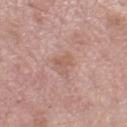follow-up: total-body-photography surveillance lesion; no biopsy
subject: female, approximately 50 years of age
tile lighting: white-light illumination
location: the leg
automated lesion analysis: a mean CIELAB color near L≈58 a*≈21 b*≈26 and a normalized border contrast of about 5; border irregularity of about 5 on a 0–10 scale and a peripheral color-asymmetry measure near 0.5; an automated nevus-likeness rating near 0 out of 100 and a lesion-detection confidence of about 100/100
image source: total-body-photography crop, ~15 mm field of view
lesion diameter: ≈2.5 mm